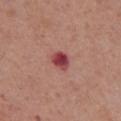{"site": "chest", "lesion_size": {"long_diameter_mm_approx": 2.5}, "image": {"source": "total-body photography crop", "field_of_view_mm": 15}, "automated_metrics": {"area_mm2_approx": 4.5, "eccentricity": 0.55, "shape_asymmetry": 0.2, "cielab_L": 44, "cielab_a": 33, "cielab_b": 21, "nevus_likeness_0_100": 0, "lesion_detection_confidence_0_100": 100}, "patient": {"sex": "male", "age_approx": 70}, "lighting": "white-light"}